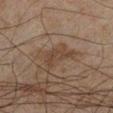Impression: Part of a total-body skin-imaging series; this lesion was reviewed on a skin check and was not flagged for biopsy. Image and clinical context: The lesion's longest dimension is about 4 mm. A male patient aged 43 to 47. Located on the left lower leg. An algorithmic analysis of the crop reported a lesion area of about 5.5 mm², a shape eccentricity near 0.9, and two-axis asymmetry of about 0.55. It also reported an average lesion color of about L≈33 a*≈13 b*≈22 (CIELAB), roughly 5 lightness units darker than nearby skin, and a lesion-to-skin contrast of about 5.5 (normalized; higher = more distinct). It also reported a border-irregularity index near 6/10, a within-lesion color-variation index near 1.5/10, and radial color variation of about 0.5. The software also gave a classifier nevus-likeness of about 0/100 and lesion-presence confidence of about 90/100. A 15 mm crop from a total-body photograph taken for skin-cancer surveillance. Imaged with cross-polarized lighting.